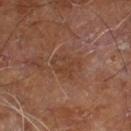Recorded during total-body skin imaging; not selected for excision or biopsy.
On the right leg.
The recorded lesion diameter is about 3 mm.
The subject is a male in their 60s.
A 15 mm crop from a total-body photograph taken for skin-cancer surveillance.